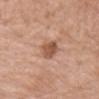Part of a total-body skin-imaging series; this lesion was reviewed on a skin check and was not flagged for biopsy.
The patient is a female aged 68–72.
A 15 mm close-up extracted from a 3D total-body photography capture.
The total-body-photography lesion software estimated a lesion area of about 6 mm², an eccentricity of roughly 0.7, and two-axis asymmetry of about 0.25. It also reported border irregularity of about 2.5 on a 0–10 scale and a within-lesion color-variation index near 3.5/10. The software also gave a classifier nevus-likeness of about 55/100 and lesion-presence confidence of about 100/100.
Located on the chest.